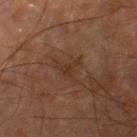follow-up: total-body-photography surveillance lesion; no biopsy | site: the right thigh | patient: male, aged 63 to 67 | diameter: ~3.5 mm (longest diameter) | lighting: cross-polarized illumination | automated metrics: a footprint of about 3.5 mm², an outline eccentricity of about 0.6 (0 = round, 1 = elongated), and a shape-asymmetry score of about 0.75 (0 = symmetric) | acquisition: 15 mm crop, total-body photography.